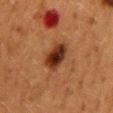Q: Was a biopsy performed?
A: imaged on a skin check; not biopsied
Q: What is the imaging modality?
A: total-body-photography crop, ~15 mm field of view
Q: Where on the body is the lesion?
A: the mid back
Q: What are the patient's age and sex?
A: female, roughly 50 years of age